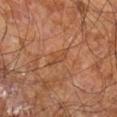Q: Was this lesion biopsied?
A: no biopsy performed (imaged during a skin exam)
Q: How was the tile lit?
A: cross-polarized illumination
Q: How large is the lesion?
A: ≈3 mm
Q: Where on the body is the lesion?
A: the right leg
Q: What is the imaging modality?
A: ~15 mm tile from a whole-body skin photo
Q: Automated lesion metrics?
A: an area of roughly 2.5 mm², a shape eccentricity near 0.9, and two-axis asymmetry of about 0.5; a lesion color around L≈45 a*≈23 b*≈33 in CIELAB, about 6 CIELAB-L* units darker than the surrounding skin, and a normalized border contrast of about 5
Q: What are the patient's age and sex?
A: male, aged approximately 60